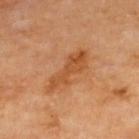This lesion was catalogued during total-body skin photography and was not selected for biopsy. Automated image analysis of the tile measured a lesion area of about 9.5 mm² and a shape eccentricity near 0.95. The analysis additionally found a nevus-likeness score of about 0/100 and a detector confidence of about 100 out of 100 that the crop contains a lesion. Cropped from a total-body skin-imaging series; the visible field is about 15 mm. The recorded lesion diameter is about 6 mm. The lesion is located on the upper back. The subject is a male in their 70s. Captured under cross-polarized illumination.